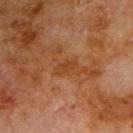Captured during whole-body skin photography for melanoma surveillance; the lesion was not biopsied. Automated tile analysis of the lesion measured an area of roughly 3 mm², a shape eccentricity near 0.85, and two-axis asymmetry of about 0.3. The software also gave roughly 5 lightness units darker than nearby skin. And it measured lesion-presence confidence of about 100/100. A close-up tile cropped from a whole-body skin photograph, about 15 mm across. A male subject aged 78 to 82. Approximately 2.5 mm at its widest. From the chest.